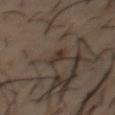– workup · no biopsy performed (imaged during a skin exam)
– diameter · ~2.5 mm (longest diameter)
– image · ~15 mm crop, total-body skin-cancer survey
– location · the chest
– subject · male, aged approximately 55
– automated metrics · a lesion-to-skin contrast of about 7.5 (normalized; higher = more distinct)